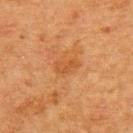Assessment: The lesion was tiled from a total-body skin photograph and was not biopsied. Acquisition and patient details: Located on the upper back. A 15 mm close-up extracted from a 3D total-body photography capture. The patient is a female approximately 50 years of age. The lesion's longest dimension is about 3 mm. This is a cross-polarized tile.